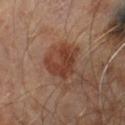Impression:
Recorded during total-body skin imaging; not selected for excision or biopsy.